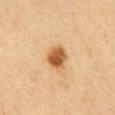Image and clinical context:
Located on the mid back. Automated image analysis of the tile measured a lesion color around L≈51 a*≈20 b*≈37 in CIELAB and about 13 CIELAB-L* units darker than the surrounding skin. The software also gave a border-irregularity index near 1/10 and a peripheral color-asymmetry measure near 1.5. The software also gave a nevus-likeness score of about 100/100. A female subject approximately 70 years of age. Imaged with cross-polarized lighting. The lesion's longest dimension is about 3.5 mm. Cropped from a total-body skin-imaging series; the visible field is about 15 mm.